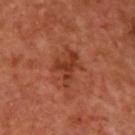Acquisition and patient details: This is a cross-polarized tile. From the upper back. A lesion tile, about 15 mm wide, cut from a 3D total-body photograph. About 4 mm across. The patient is a male aged approximately 50. Automated tile analysis of the lesion measured a lesion color around L≈38 a*≈28 b*≈34 in CIELAB and roughly 9 lightness units darker than nearby skin. The software also gave a border-irregularity rating of about 6.5/10, internal color variation of about 2 on a 0–10 scale, and a peripheral color-asymmetry measure near 0.5.